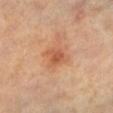- workup · total-body-photography surveillance lesion; no biopsy
- location · the left lower leg
- diameter · ~2.5 mm (longest diameter)
- illumination · cross-polarized
- image · total-body-photography crop, ~15 mm field of view
- subject · female, about 65 years old